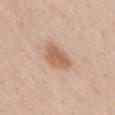Captured under white-light illumination.
A male patient, about 60 years old.
From the lower back.
Longest diameter approximately 4 mm.
A 15 mm close-up tile from a total-body photography series done for melanoma screening.